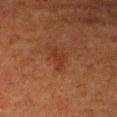Assessment:
Captured during whole-body skin photography for melanoma surveillance; the lesion was not biopsied.
Context:
Longest diameter approximately 3 mm. A 15 mm crop from a total-body photograph taken for skin-cancer surveillance. Captured under cross-polarized illumination. A female patient aged around 50. Located on the right upper arm. Automated tile analysis of the lesion measured a lesion area of about 3 mm², an outline eccentricity of about 0.85 (0 = round, 1 = elongated), and a shape-asymmetry score of about 0.65 (0 = symmetric). And it measured a nevus-likeness score of about 5/100 and a lesion-detection confidence of about 100/100.